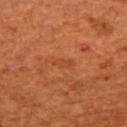Findings:
• follow-up: total-body-photography surveillance lesion; no biopsy
• image: total-body-photography crop, ~15 mm field of view
• site: the upper back
• patient: female, aged around 65